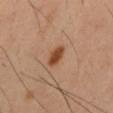Part of a total-body skin-imaging series; this lesion was reviewed on a skin check and was not flagged for biopsy.